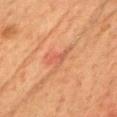Clinical impression: Part of a total-body skin-imaging series; this lesion was reviewed on a skin check and was not flagged for biopsy. Context: On the head or neck. A female patient, in their mid- to late 50s. A 15 mm close-up tile from a total-body photography series done for melanoma screening. The recorded lesion diameter is about 3 mm. The total-body-photography lesion software estimated a lesion area of about 3 mm², an outline eccentricity of about 0.85 (0 = round, 1 = elongated), and a symmetry-axis asymmetry near 0.45. The software also gave a classifier nevus-likeness of about 5/100. Captured under cross-polarized illumination.